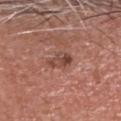Findings:
* image source · 15 mm crop, total-body photography
* location · the head or neck
* subject · male, aged 58–62